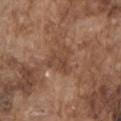Case summary:
– biopsy status — imaged on a skin check; not biopsied
– body site — the left upper arm
– lighting — white-light illumination
– patient — male, approximately 75 years of age
– acquisition — 15 mm crop, total-body photography